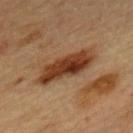No biopsy was performed on this lesion — it was imaged during a full skin examination and was not determined to be concerning. Automated tile analysis of the lesion measured an average lesion color of about L≈34 a*≈20 b*≈30 (CIELAB), roughly 13 lightness units darker than nearby skin, and a lesion-to-skin contrast of about 11.5 (normalized; higher = more distinct). A female patient, about 60 years old. The lesion is located on the back. A 15 mm close-up extracted from a 3D total-body photography capture. This is a cross-polarized tile.